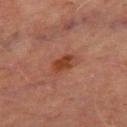Imaged during a routine full-body skin examination; the lesion was not biopsied and no histopathology is available. A male patient aged around 70. This is a cross-polarized tile. The recorded lesion diameter is about 3.5 mm. From the left thigh. A close-up tile cropped from a whole-body skin photograph, about 15 mm across.